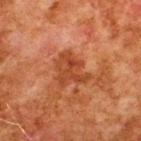workup: total-body-photography surveillance lesion; no biopsy | image: ~15 mm tile from a whole-body skin photo | lighting: cross-polarized illumination | patient: male, aged approximately 80 | lesion diameter: about 4 mm | anatomic site: the upper back.